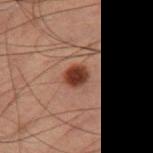biopsy_status: not biopsied; imaged during a skin examination
image:
  source: total-body photography crop
  field_of_view_mm: 15
lighting: cross-polarized
site: leg
automated_metrics:
  border_irregularity_0_10: 1.5
  color_variation_0_10: 3.5
  peripheral_color_asymmetry: 1.0
lesion_size:
  long_diameter_mm_approx: 2.5
patient:
  sex: male
  age_approx: 60